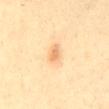biopsy status: no biopsy performed (imaged during a skin exam)
acquisition: ~15 mm tile from a whole-body skin photo
lesion size: about 2.5 mm
subject: female, in their mid-40s
location: the mid back